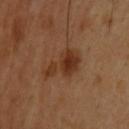Q: Is there a histopathology result?
A: no biopsy performed (imaged during a skin exam)
Q: How was this image acquired?
A: ~15 mm crop, total-body skin-cancer survey
Q: Lesion location?
A: the upper back
Q: Who is the patient?
A: male, roughly 65 years of age